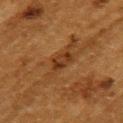Findings:
- workup · no biopsy performed (imaged during a skin exam)
- location · the left upper arm
- size · ≈3 mm
- patient · female, approximately 50 years of age
- acquisition · ~15 mm crop, total-body skin-cancer survey
- lighting · cross-polarized